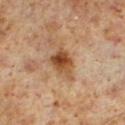Imaged during a routine full-body skin examination; the lesion was not biopsied and no histopathology is available. A male patient about 60 years old. Automated image analysis of the tile measured a footprint of about 8.5 mm², an eccentricity of roughly 0.75, and a symmetry-axis asymmetry near 0.35. And it measured an automated nevus-likeness rating near 90 out of 100 and lesion-presence confidence of about 100/100. The lesion's longest dimension is about 4.5 mm. A 15 mm close-up extracted from a 3D total-body photography capture. The lesion is located on the leg.